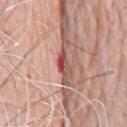{
  "lighting": "white-light",
  "site": "chest",
  "automated_metrics": {
    "area_mm2_approx": 3.5,
    "shape_asymmetry": 0.35,
    "border_irregularity_0_10": 4.0,
    "color_variation_0_10": 5.5,
    "peripheral_color_asymmetry": 1.5
  },
  "patient": {
    "sex": "male",
    "age_approx": 80
  },
  "image": {
    "source": "total-body photography crop",
    "field_of_view_mm": 15
  },
  "lesion_size": {
    "long_diameter_mm_approx": 3.0
  }
}